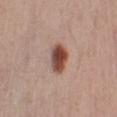Clinical summary:
This is a white-light tile. The lesion is on the left upper arm. A male subject aged around 55. Longest diameter approximately 3.5 mm. Cropped from a whole-body photographic skin survey; the tile spans about 15 mm. The lesion-visualizer software estimated an outline eccentricity of about 0.7 (0 = round, 1 = elongated) and a symmetry-axis asymmetry near 0.15. It also reported a lesion-detection confidence of about 100/100.